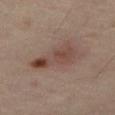No biopsy was performed on this lesion — it was imaged during a full skin examination and was not determined to be concerning. A male subject in their mid-60s. On the right thigh. A 15 mm close-up extracted from a 3D total-body photography capture.